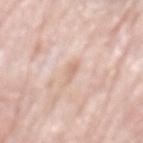| feature | finding |
|---|---|
| notes | catalogued during a skin exam; not biopsied |
| image | ~15 mm crop, total-body skin-cancer survey |
| location | the mid back |
| subject | male, aged 78–82 |
| tile lighting | white-light |